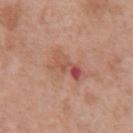subject: male, aged around 70 | tile lighting: white-light | anatomic site: the front of the torso | image source: ~15 mm crop, total-body skin-cancer survey | TBP lesion metrics: a border-irregularity rating of about 5/10, a within-lesion color-variation index near 10/10, and a peripheral color-asymmetry measure near 3.5 | lesion diameter: ~4.5 mm (longest diameter).